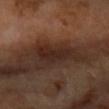  biopsy_status: not biopsied; imaged during a skin examination
  patient:
    sex: female
    age_approx: 60
  image:
    source: total-body photography crop
    field_of_view_mm: 15
  site: right forearm